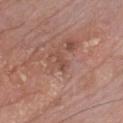| feature | finding |
|---|---|
| lighting | white-light illumination |
| image source | ~15 mm crop, total-body skin-cancer survey |
| automated metrics | a lesion–skin lightness drop of about 7 and a lesion-to-skin contrast of about 5 (normalized; higher = more distinct); a border-irregularity index near 4/10 and internal color variation of about 0.5 on a 0–10 scale |
| subject | male, aged 58 to 62 |
| diameter | about 2.5 mm |
| location | the front of the torso |